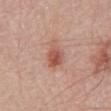{"biopsy_status": "not biopsied; imaged during a skin examination", "lighting": "white-light", "patient": {"sex": "male", "age_approx": 70}, "image": {"source": "total-body photography crop", "field_of_view_mm": 15}, "automated_metrics": {"nevus_likeness_0_100": 85, "lesion_detection_confidence_0_100": 100}, "site": "back", "lesion_size": {"long_diameter_mm_approx": 4.5}}